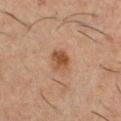  biopsy_status: not biopsied; imaged during a skin examination
  site: chest
  lighting: cross-polarized
  lesion_size:
    long_diameter_mm_approx: 3.0
  automated_metrics:
    area_mm2_approx: 6.0
    eccentricity: 0.6
    color_variation_0_10: 3.5
    peripheral_color_asymmetry: 1.0
    nevus_likeness_0_100: 85
  patient:
    sex: male
    age_approx: 50
  image:
    source: total-body photography crop
    field_of_view_mm: 15